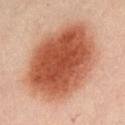No biopsy was performed on this lesion — it was imaged during a full skin examination and was not determined to be concerning. Located on the abdomen. The tile uses cross-polarized illumination. The lesion-visualizer software estimated a color-variation rating of about 7/10 and peripheral color asymmetry of about 2. The software also gave a classifier nevus-likeness of about 100/100 and lesion-presence confidence of about 100/100. A female patient in their mid-30s. Cropped from a total-body skin-imaging series; the visible field is about 15 mm. Approximately 11 mm at its widest.